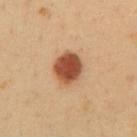The lesion was tiled from a total-body skin photograph and was not biopsied.